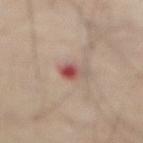Assessment:
Captured during whole-body skin photography for melanoma surveillance; the lesion was not biopsied.
Acquisition and patient details:
A region of skin cropped from a whole-body photographic capture, roughly 15 mm wide. Located on the abdomen. The subject is a male aged 68 to 72.